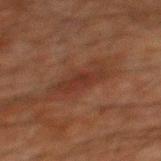Q: Is there a histopathology result?
A: catalogued during a skin exam; not biopsied